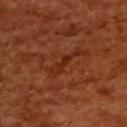Clinical impression: Imaged during a routine full-body skin examination; the lesion was not biopsied and no histopathology is available. Background: The lesion is located on the back. A 15 mm close-up extracted from a 3D total-body photography capture. A female subject, about 55 years old. The total-body-photography lesion software estimated a border-irregularity rating of about 5/10, a color-variation rating of about 0/10, and a peripheral color-asymmetry measure near 0. The lesion's longest dimension is about 3 mm.